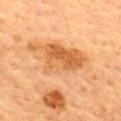| feature | finding |
|---|---|
| notes | catalogued during a skin exam; not biopsied |
| lighting | cross-polarized illumination |
| site | the upper back |
| TBP lesion metrics | a nevus-likeness score of about 15/100 |
| image | 15 mm crop, total-body photography |
| patient | female, roughly 55 years of age |
| lesion size | ~10.5 mm (longest diameter) |